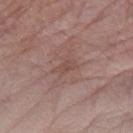Case summary:
- site: the left forearm
- imaging modality: ~15 mm tile from a whole-body skin photo
- subject: female, approximately 65 years of age